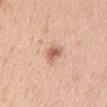Findings:
– biopsy status · no biopsy performed (imaged during a skin exam)
– lighting · white-light illumination
– acquisition · total-body-photography crop, ~15 mm field of view
– patient · female, about 50 years old
– lesion size · ≈3 mm
– anatomic site · the back
– automated metrics · a lesion color around L≈61 a*≈22 b*≈32 in CIELAB and a normalized lesion–skin contrast near 8